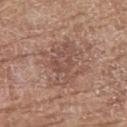Findings:
– biopsy status · no biopsy performed (imaged during a skin exam)
– lesion diameter · about 5.5 mm
– automated metrics · a border-irregularity rating of about 6.5/10, internal color variation of about 4 on a 0–10 scale, and peripheral color asymmetry of about 1.5
– patient · female, aged approximately 75
– tile lighting · white-light illumination
– image · 15 mm crop, total-body photography
– location · the left thigh